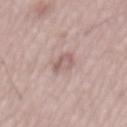follow-up — total-body-photography surveillance lesion; no biopsy | subject — male, approximately 55 years of age | acquisition — total-body-photography crop, ~15 mm field of view | lighting — white-light illumination | diameter — ~3.5 mm (longest diameter).